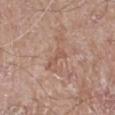Impression: Captured during whole-body skin photography for melanoma surveillance; the lesion was not biopsied. Context: The lesion's longest dimension is about 2.5 mm. A male subject, aged approximately 80. A 15 mm crop from a total-body photograph taken for skin-cancer surveillance. From the right lower leg.